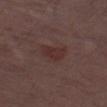Imaged during a routine full-body skin examination; the lesion was not biopsied and no histopathology is available.
A male subject, approximately 70 years of age.
Longest diameter approximately 3 mm.
On the left thigh.
Imaged with white-light lighting.
Cropped from a total-body skin-imaging series; the visible field is about 15 mm.